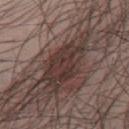Q: Is there a histopathology result?
A: catalogued during a skin exam; not biopsied